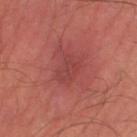follow-up: total-body-photography surveillance lesion; no biopsy | site: the leg | patient: male, in their mid-30s | imaging modality: ~15 mm tile from a whole-body skin photo.